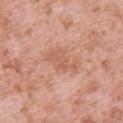Image and clinical context: Approximately 4 mm at its widest. The patient is a female roughly 40 years of age. A 15 mm crop from a total-body photograph taken for skin-cancer surveillance. On the back. Automated tile analysis of the lesion measured a lesion area of about 6.5 mm². The software also gave an average lesion color of about L≈60 a*≈24 b*≈32 (CIELAB), roughly 7 lightness units darker than nearby skin, and a normalized lesion–skin contrast near 5. The analysis additionally found a color-variation rating of about 2/10 and a peripheral color-asymmetry measure near 0.5. It also reported a nevus-likeness score of about 0/100 and lesion-presence confidence of about 100/100.